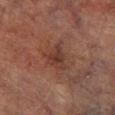The lesion was tiled from a total-body skin photograph and was not biopsied. From the right lower leg. The tile uses cross-polarized illumination. A roughly 15 mm field-of-view crop from a total-body skin photograph. The patient is a male in their mid- to late 70s. Automated tile analysis of the lesion measured a footprint of about 6 mm², an eccentricity of roughly 0.7, and a symmetry-axis asymmetry near 0.4.